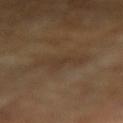  image:
    source: total-body photography crop
    field_of_view_mm: 15
  lighting: cross-polarized
  lesion_size:
    long_diameter_mm_approx: 5.5
  site: right forearm
  automated_metrics:
    border_irregularity_0_10: 5.5
    color_variation_0_10: 1.5
    peripheral_color_asymmetry: 0.5
  patient:
    sex: male
    age_approx: 85+